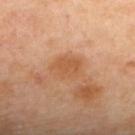  lesion_size:
    long_diameter_mm_approx: 3.5
  lighting: cross-polarized
  site: upper back
  image:
    source: total-body photography crop
    field_of_view_mm: 15
  patient:
    sex: female
    age_approx: 45